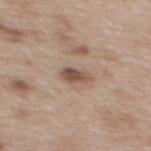Q: Is there a histopathology result?
A: imaged on a skin check; not biopsied
Q: What is the lesion's diameter?
A: about 3 mm
Q: What is the imaging modality?
A: 15 mm crop, total-body photography
Q: How was the tile lit?
A: white-light
Q: What are the patient's age and sex?
A: female, roughly 40 years of age
Q: Lesion location?
A: the back